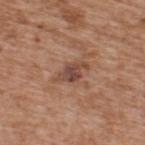This is a white-light tile. A male patient, approximately 65 years of age. The lesion's longest dimension is about 3.5 mm. A region of skin cropped from a whole-body photographic capture, roughly 15 mm wide. The lesion is on the back.